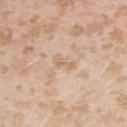Part of a total-body skin-imaging series; this lesion was reviewed on a skin check and was not flagged for biopsy. A female patient aged 23 to 27. Captured under white-light illumination. Approximately 3 mm at its widest. The lesion is on the arm. The lesion-visualizer software estimated a mean CIELAB color near L≈66 a*≈17 b*≈35, a lesion–skin lightness drop of about 7, and a lesion-to-skin contrast of about 5.5 (normalized; higher = more distinct). Cropped from a total-body skin-imaging series; the visible field is about 15 mm.